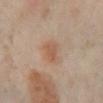The lesion was tiled from a total-body skin photograph and was not biopsied.